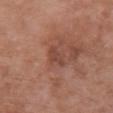Assessment:
The lesion was photographed on a routine skin check and not biopsied; there is no pathology result.
Acquisition and patient details:
Cropped from a total-body skin-imaging series; the visible field is about 15 mm. From the chest. Automated tile analysis of the lesion measured a lesion area of about 3 mm², an eccentricity of roughly 0.85, and two-axis asymmetry of about 0.35. The analysis additionally found a lesion color around L≈44 a*≈23 b*≈27 in CIELAB and a normalized border contrast of about 6. The software also gave a classifier nevus-likeness of about 0/100 and lesion-presence confidence of about 100/100. A female patient, aged 68 to 72. Measured at roughly 3 mm in maximum diameter.